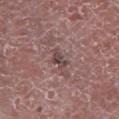Part of a total-body skin-imaging series; this lesion was reviewed on a skin check and was not flagged for biopsy. The lesion's longest dimension is about 2.5 mm. A close-up tile cropped from a whole-body skin photograph, about 15 mm across. The patient is a male about 75 years old. The tile uses white-light illumination. On the left lower leg.